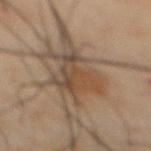Assessment: Captured during whole-body skin photography for melanoma surveillance; the lesion was not biopsied. Clinical summary: Imaged with cross-polarized lighting. About 6 mm across. A region of skin cropped from a whole-body photographic capture, roughly 15 mm wide. The lesion is located on the abdomen. A male subject aged approximately 50.